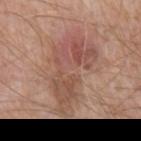Captured during whole-body skin photography for melanoma surveillance; the lesion was not biopsied. Approximately 10 mm at its widest. A lesion tile, about 15 mm wide, cut from a 3D total-body photograph. The lesion is located on the mid back. The subject is a male roughly 60 years of age. Automated tile analysis of the lesion measured a footprint of about 33 mm², an outline eccentricity of about 0.9 (0 = round, 1 = elongated), and a shape-asymmetry score of about 0.35 (0 = symmetric). The software also gave a mean CIELAB color near L≈52 a*≈21 b*≈26, a lesion–skin lightness drop of about 9, and a normalized border contrast of about 6. This is a white-light tile.